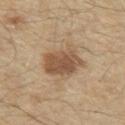image: ~15 mm tile from a whole-body skin photo
automated metrics: an area of roughly 13 mm², an eccentricity of roughly 0.75, and a shape-asymmetry score of about 0.2 (0 = symmetric); an average lesion color of about L≈52 a*≈17 b*≈32 (CIELAB) and about 12 CIELAB-L* units darker than the surrounding skin; a within-lesion color-variation index near 4/10 and a peripheral color-asymmetry measure near 1.5; a classifier nevus-likeness of about 75/100
location: the chest
subject: male, aged 68 to 72
lighting: white-light
diameter: about 5 mm